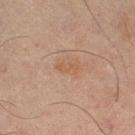Impression: Part of a total-body skin-imaging series; this lesion was reviewed on a skin check and was not flagged for biopsy. Context: A close-up tile cropped from a whole-body skin photograph, about 15 mm across. A male subject, aged approximately 65. Captured under cross-polarized illumination. Longest diameter approximately 2.5 mm. On the left thigh.